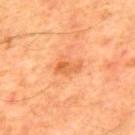This lesion was catalogued during total-body skin photography and was not selected for biopsy.
On the mid back.
A lesion tile, about 15 mm wide, cut from a 3D total-body photograph.
A male subject roughly 65 years of age.